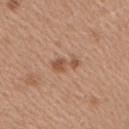Imaged during a routine full-body skin examination; the lesion was not biopsied and no histopathology is available. A female patient aged 38 to 42. Imaged with white-light lighting. About 3.5 mm across. A region of skin cropped from a whole-body photographic capture, roughly 15 mm wide. The lesion is located on the right upper arm.